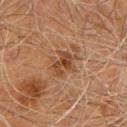The lesion was tiled from a total-body skin photograph and was not biopsied.
Measured at roughly 3.5 mm in maximum diameter.
A 15 mm crop from a total-body photograph taken for skin-cancer surveillance.
The subject is a male aged approximately 60.
The lesion is on the front of the torso.
Captured under cross-polarized illumination.